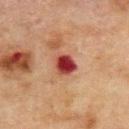Clinical summary: The lesion's longest dimension is about 3 mm. A 15 mm crop from a total-body photograph taken for skin-cancer surveillance. A male subject, aged 68–72. The lesion is on the upper back. An algorithmic analysis of the crop reported a footprint of about 6 mm², an outline eccentricity of about 0.5 (0 = round, 1 = elongated), and a symmetry-axis asymmetry near 0.2. And it measured a mean CIELAB color near L≈35 a*≈30 b*≈25, roughly 16 lightness units darker than nearby skin, and a normalized border contrast of about 13.5. It also reported border irregularity of about 1.5 on a 0–10 scale.